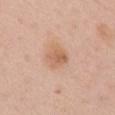{
  "image": {
    "source": "total-body photography crop",
    "field_of_view_mm": 15
  },
  "site": "mid back",
  "lesion_size": {
    "long_diameter_mm_approx": 3.0
  },
  "patient": {
    "sex": "female",
    "age_approx": 50
  },
  "lighting": "white-light"
}